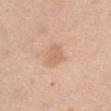Q: Was a biopsy performed?
A: catalogued during a skin exam; not biopsied
Q: Where on the body is the lesion?
A: the right thigh
Q: How was this image acquired?
A: ~15 mm crop, total-body skin-cancer survey
Q: What lighting was used for the tile?
A: white-light
Q: What did automated image analysis measure?
A: an eccentricity of roughly 0.4; a border-irregularity index near 2.5/10 and a color-variation rating of about 1.5/10; a classifier nevus-likeness of about 25/100 and lesion-presence confidence of about 100/100
Q: What are the patient's age and sex?
A: female, about 65 years old
Q: Lesion size?
A: ≈2.5 mm